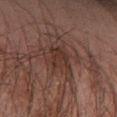workup=catalogued during a skin exam; not biopsied | imaging modality=15 mm crop, total-body photography | location=the right forearm | illumination=cross-polarized illumination | patient=male, about 70 years old | lesion size=~3.5 mm (longest diameter) | automated metrics=a shape eccentricity near 0.6 and a symmetry-axis asymmetry near 0.3; an average lesion color of about L≈26 a*≈15 b*≈19 (CIELAB), roughly 6 lightness units darker than nearby skin, and a normalized border contrast of about 6.5; a nevus-likeness score of about 0/100 and a detector confidence of about 80 out of 100 that the crop contains a lesion.